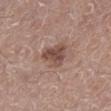Case summary:
• follow-up — catalogued during a skin exam; not biopsied
• TBP lesion metrics — a footprint of about 7.5 mm², an outline eccentricity of about 0.65 (0 = round, 1 = elongated), and a shape-asymmetry score of about 0.45 (0 = symmetric); a lesion color around L≈48 a*≈19 b*≈24 in CIELAB and a lesion–skin lightness drop of about 11; an automated nevus-likeness rating near 30 out of 100
• body site — the leg
• size — about 3.5 mm
• illumination — white-light
• subject — male, aged around 60
• image source — ~15 mm tile from a whole-body skin photo